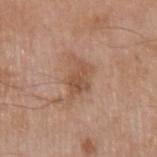{"lighting": "white-light", "image": {"source": "total-body photography crop", "field_of_view_mm": 15}, "site": "left upper arm", "lesion_size": {"long_diameter_mm_approx": 4.5}, "automated_metrics": {"eccentricity": 0.75, "border_irregularity_0_10": 5.0, "color_variation_0_10": 3.0, "peripheral_color_asymmetry": 1.0, "nevus_likeness_0_100": 5, "lesion_detection_confidence_0_100": 100}, "patient": {"sex": "male", "age_approx": 65}}